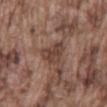| feature | finding |
|---|---|
| workup | no biopsy performed (imaged during a skin exam) |
| imaging modality | 15 mm crop, total-body photography |
| lesion size | ≈3.5 mm |
| TBP lesion metrics | an area of roughly 7.5 mm²; a lesion color around L≈42 a*≈18 b*≈23 in CIELAB, about 10 CIELAB-L* units darker than the surrounding skin, and a normalized border contrast of about 8; a border-irregularity index near 3.5/10 and radial color variation of about 1 |
| tile lighting | white-light |
| site | the mid back |
| patient | male, in their mid- to late 70s |